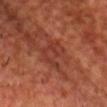{"biopsy_status": "not biopsied; imaged during a skin examination", "site": "chest", "automated_metrics": {"eccentricity": 0.75, "shape_asymmetry": 0.55, "peripheral_color_asymmetry": 0.5, "nevus_likeness_0_100": 0, "lesion_detection_confidence_0_100": 60}, "patient": {"sex": "male", "age_approx": 60}, "lesion_size": {"long_diameter_mm_approx": 4.5}, "image": {"source": "total-body photography crop", "field_of_view_mm": 15}, "lighting": "cross-polarized"}